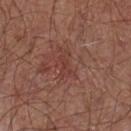Assessment:
No biopsy was performed on this lesion — it was imaged during a full skin examination and was not determined to be concerning.
Background:
Approximately 3 mm at its widest. A male subject roughly 55 years of age. This image is a 15 mm lesion crop taken from a total-body photograph. The lesion is located on the front of the torso. The tile uses white-light illumination.